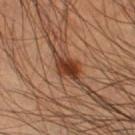The lesion was tiled from a total-body skin photograph and was not biopsied.
A male subject, aged 43 to 47.
A 15 mm close-up extracted from a 3D total-body photography capture.
On the right thigh.
The tile uses cross-polarized illumination.
The lesion-visualizer software estimated an area of roughly 5.5 mm² and an outline eccentricity of about 0.85 (0 = round, 1 = elongated). The software also gave about 13 CIELAB-L* units darker than the surrounding skin and a normalized border contrast of about 11.5. The software also gave a border-irregularity index near 4.5/10, a color-variation rating of about 3/10, and radial color variation of about 1.
Longest diameter approximately 4 mm.